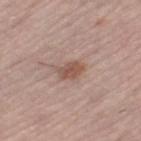Notes:
* notes · catalogued during a skin exam; not biopsied
* image · 15 mm crop, total-body photography
* site · the leg
* subject · female, aged 63 to 67